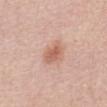Notes:
– notes — catalogued during a skin exam; not biopsied
– anatomic site — the abdomen
– imaging modality — total-body-photography crop, ~15 mm field of view
– patient — female, roughly 60 years of age
– illumination — white-light illumination